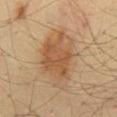Imaged during a routine full-body skin examination; the lesion was not biopsied and no histopathology is available. The subject is a male in their mid-30s. Measured at roughly 7.5 mm in maximum diameter. The lesion is on the mid back. Imaged with cross-polarized lighting. This image is a 15 mm lesion crop taken from a total-body photograph.